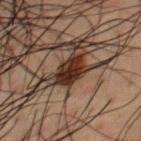On the chest.
A male patient aged 48–52.
A close-up tile cropped from a whole-body skin photograph, about 15 mm across.
Captured under cross-polarized illumination.